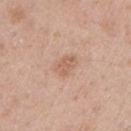  image:
    source: total-body photography crop
    field_of_view_mm: 15
  lighting: white-light
  patient:
    sex: male
    age_approx: 55
  site: left upper arm
  lesion_size:
    long_diameter_mm_approx: 3.0